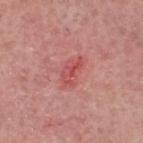Imaged during a routine full-body skin examination; the lesion was not biopsied and no histopathology is available. A roughly 15 mm field-of-view crop from a total-body skin photograph. On the head or neck. A male patient aged 73–77. Captured under white-light illumination.